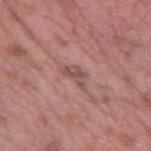Assessment: Captured during whole-body skin photography for melanoma surveillance; the lesion was not biopsied. Background: A male subject aged 38 to 42. A close-up tile cropped from a whole-body skin photograph, about 15 mm across. Captured under white-light illumination. On the right upper arm.